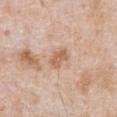notes: total-body-photography surveillance lesion; no biopsy
illumination: white-light
automated lesion analysis: a footprint of about 4.5 mm², a shape eccentricity near 0.75, and a shape-asymmetry score of about 0.25 (0 = symmetric); border irregularity of about 2.5 on a 0–10 scale, a color-variation rating of about 2/10, and peripheral color asymmetry of about 1
location: the abdomen
acquisition: ~15 mm tile from a whole-body skin photo
patient: male, aged 63 to 67
size: ≈2.5 mm